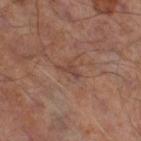subject: male, aged 63 to 67 | automated metrics: a footprint of about 2.5 mm², an outline eccentricity of about 0.85 (0 = round, 1 = elongated), and a shape-asymmetry score of about 0.6 (0 = symmetric); a mean CIELAB color near L≈40 a*≈19 b*≈23, roughly 6 lightness units darker than nearby skin, and a normalized border contrast of about 5.5 | site: the right thigh | size: ~2.5 mm (longest diameter) | imaging modality: ~15 mm crop, total-body skin-cancer survey.